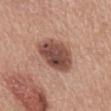biopsy status=total-body-photography surveillance lesion; no biopsy
site=the back
illumination=white-light
lesion size=~5 mm (longest diameter)
image-analysis metrics=a border-irregularity index near 1.5/10 and a peripheral color-asymmetry measure near 2
image=~15 mm tile from a whole-body skin photo
patient=male, in their mid-50s